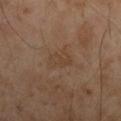No biopsy was performed on this lesion — it was imaged during a full skin examination and was not determined to be concerning.
Automated tile analysis of the lesion measured an area of roughly 6 mm², an outline eccentricity of about 0.8 (0 = round, 1 = elongated), and two-axis asymmetry of about 0.25. The software also gave an average lesion color of about L≈41 a*≈16 b*≈29 (CIELAB), roughly 5 lightness units darker than nearby skin, and a lesion-to-skin contrast of about 4.5 (normalized; higher = more distinct). The analysis additionally found a lesion-detection confidence of about 100/100.
A male patient, about 55 years old.
Longest diameter approximately 3.5 mm.
The lesion is on the left upper arm.
A 15 mm close-up tile from a total-body photography series done for melanoma screening.